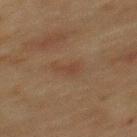subject = male, aged 48–52
illumination = cross-polarized
automated metrics = an area of roughly 3 mm², an eccentricity of roughly 0.85, and two-axis asymmetry of about 0.5; a border-irregularity rating of about 5.5/10, internal color variation of about 1 on a 0–10 scale, and peripheral color asymmetry of about 0; an automated nevus-likeness rating near 0 out of 100 and a lesion-detection confidence of about 100/100
image = ~15 mm crop, total-body skin-cancer survey
anatomic site = the mid back